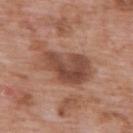follow-up: catalogued during a skin exam; not biopsied | imaging modality: ~15 mm crop, total-body skin-cancer survey | lighting: white-light illumination | patient: male, aged around 60 | diameter: about 7.5 mm | site: the upper back.